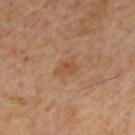{
  "biopsy_status": "not biopsied; imaged during a skin examination",
  "lighting": "cross-polarized",
  "image": {
    "source": "total-body photography crop",
    "field_of_view_mm": 15
  },
  "lesion_size": {
    "long_diameter_mm_approx": 3.0
  },
  "patient": {
    "sex": "male",
    "age_approx": 60
  },
  "site": "mid back"
}